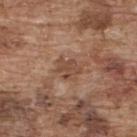Captured during whole-body skin photography for melanoma surveillance; the lesion was not biopsied.
Located on the upper back.
Automated tile analysis of the lesion measured an eccentricity of roughly 0.6 and a symmetry-axis asymmetry near 0.4. And it measured a mean CIELAB color near L≈47 a*≈20 b*≈29, roughly 8 lightness units darker than nearby skin, and a lesion-to-skin contrast of about 6.5 (normalized; higher = more distinct). The software also gave a border-irregularity index near 4.5/10, internal color variation of about 2 on a 0–10 scale, and a peripheral color-asymmetry measure near 1. The software also gave a nevus-likeness score of about 0/100 and lesion-presence confidence of about 100/100.
This image is a 15 mm lesion crop taken from a total-body photograph.
A male subject, approximately 75 years of age.
Captured under white-light illumination.